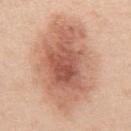- notes · total-body-photography surveillance lesion; no biopsy
- site · the abdomen
- diameter · about 12.5 mm
- automated lesion analysis · a lesion–skin lightness drop of about 13; border irregularity of about 2.5 on a 0–10 scale, a color-variation rating of about 7.5/10, and radial color variation of about 2
- subject · male, in their mid- to late 40s
- imaging modality · ~15 mm crop, total-body skin-cancer survey
- lighting · white-light illumination